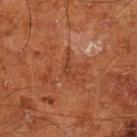No biopsy was performed on this lesion — it was imaged during a full skin examination and was not determined to be concerning.